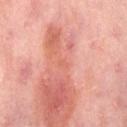Impression:
Captured during whole-body skin photography for melanoma surveillance; the lesion was not biopsied.
Acquisition and patient details:
Located on the abdomen. Measured at roughly 1.5 mm in maximum diameter. A region of skin cropped from a whole-body photographic capture, roughly 15 mm wide. A female subject aged 48–52.